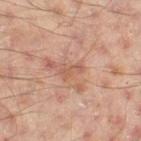Clinical impression:
Recorded during total-body skin imaging; not selected for excision or biopsy.
Background:
A 15 mm crop from a total-body photograph taken for skin-cancer surveillance. Measured at roughly 3 mm in maximum diameter. Located on the left thigh. A male subject aged 43 to 47.